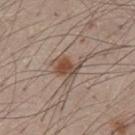This lesion was catalogued during total-body skin photography and was not selected for biopsy. The lesion's longest dimension is about 3.5 mm. The lesion is on the front of the torso. Cropped from a total-body skin-imaging series; the visible field is about 15 mm. The tile uses white-light illumination. The patient is a male about 55 years old.